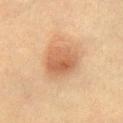notes: no biopsy performed (imaged during a skin exam)
acquisition: ~15 mm tile from a whole-body skin photo
patient: male, roughly 60 years of age
lesion size: ~4 mm (longest diameter)
body site: the lower back